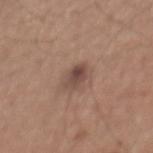The lesion was tiled from a total-body skin photograph and was not biopsied. This image is a 15 mm lesion crop taken from a total-body photograph. The subject is a male aged 63 to 67. The lesion is located on the mid back. Approximately 3 mm at its widest. This is a white-light tile.